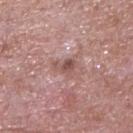Q: Is there a histopathology result?
A: imaged on a skin check; not biopsied
Q: What kind of image is this?
A: ~15 mm crop, total-body skin-cancer survey
Q: What are the patient's age and sex?
A: male, in their mid- to late 60s
Q: What is the lesion's diameter?
A: ≈3 mm
Q: Lesion location?
A: the upper back